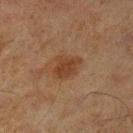Assessment: Imaged during a routine full-body skin examination; the lesion was not biopsied and no histopathology is available. Image and clinical context: The total-body-photography lesion software estimated a nevus-likeness score of about 75/100. A 15 mm crop from a total-body photograph taken for skin-cancer surveillance. The tile uses cross-polarized illumination. From the right lower leg. Longest diameter approximately 3.5 mm. A male patient aged 63–67.